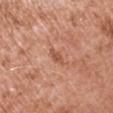Imaged during a routine full-body skin examination; the lesion was not biopsied and no histopathology is available. Cropped from a whole-body photographic skin survey; the tile spans about 15 mm. Longest diameter approximately 2.5 mm. Located on the left upper arm. The total-body-photography lesion software estimated a footprint of about 2.5 mm² and a shape-asymmetry score of about 0.35 (0 = symmetric). It also reported a lesion color around L≈54 a*≈25 b*≈34 in CIELAB, a lesion–skin lightness drop of about 9, and a lesion-to-skin contrast of about 6.5 (normalized; higher = more distinct). And it measured a classifier nevus-likeness of about 0/100 and lesion-presence confidence of about 100/100. Imaged with white-light lighting. A male subject aged 73 to 77.